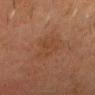<record>
<biopsy_status>not biopsied; imaged during a skin examination</biopsy_status>
<site>head or neck</site>
<patient>
  <sex>male</sex>
  <age_approx>45</age_approx>
</patient>
<lesion_size>
  <long_diameter_mm_approx>5.0</long_diameter_mm_approx>
</lesion_size>
<image>
  <source>total-body photography crop</source>
  <field_of_view_mm>15</field_of_view_mm>
</image>
<lighting>cross-polarized</lighting>
</record>